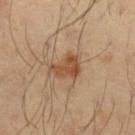patient — male, aged around 40; location — the left forearm; image source — ~15 mm crop, total-body skin-cancer survey; lesion diameter — ~3.5 mm (longest diameter).